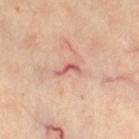Imaged during a routine full-body skin examination; the lesion was not biopsied and no histopathology is available. A region of skin cropped from a whole-body photographic capture, roughly 15 mm wide. Located on the right thigh. A female patient, in their 40s. This is a cross-polarized tile. An algorithmic analysis of the crop reported about 12 CIELAB-L* units darker than the surrounding skin and a normalized border contrast of about 8.